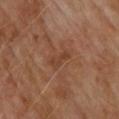follow-up: total-body-photography surveillance lesion; no biopsy | acquisition: ~15 mm crop, total-body skin-cancer survey | subject: male, aged 68–72 | body site: the upper back | diameter: ~3 mm (longest diameter).